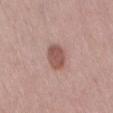Q: Was this lesion biopsied?
A: catalogued during a skin exam; not biopsied
Q: What is the anatomic site?
A: the lower back
Q: Who is the patient?
A: female, about 40 years old
Q: Automated lesion metrics?
A: a lesion area of about 7 mm², an eccentricity of roughly 0.65, and a symmetry-axis asymmetry near 0.1; border irregularity of about 1 on a 0–10 scale, a within-lesion color-variation index near 2/10, and a peripheral color-asymmetry measure near 0.5
Q: How was this image acquired?
A: 15 mm crop, total-body photography
Q: Lesion size?
A: ≈3.5 mm